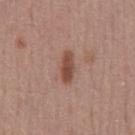Assessment: Part of a total-body skin-imaging series; this lesion was reviewed on a skin check and was not flagged for biopsy. Acquisition and patient details: This is a white-light tile. On the chest. A region of skin cropped from a whole-body photographic capture, roughly 15 mm wide. An algorithmic analysis of the crop reported a shape eccentricity near 0.9 and a shape-asymmetry score of about 0.3 (0 = symmetric). The software also gave a mean CIELAB color near L≈47 a*≈21 b*≈27, about 11 CIELAB-L* units darker than the surrounding skin, and a normalized border contrast of about 8.5. The analysis additionally found radial color variation of about 1. The analysis additionally found a classifier nevus-likeness of about 95/100. The recorded lesion diameter is about 3.5 mm. The patient is a male aged 43 to 47.